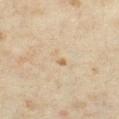The lesion was tiled from a total-body skin photograph and was not biopsied. The total-body-photography lesion software estimated a footprint of about 2 mm², an eccentricity of roughly 0.9, and a shape-asymmetry score of about 0.5 (0 = symmetric). And it measured an automated nevus-likeness rating near 0 out of 100 and lesion-presence confidence of about 100/100. The subject is a female aged 33 to 37. Captured under cross-polarized illumination. Longest diameter approximately 2.5 mm. A 15 mm close-up tile from a total-body photography series done for melanoma screening. From the right thigh.